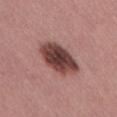Case summary:
– workup · total-body-photography surveillance lesion; no biopsy
– patient · male, about 55 years old
– body site · the lower back
– image source · ~15 mm crop, total-body skin-cancer survey